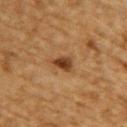Part of a total-body skin-imaging series; this lesion was reviewed on a skin check and was not flagged for biopsy. On the upper back. Approximately 2.5 mm at its widest. The subject is a male approximately 85 years of age or older. Automated tile analysis of the lesion measured a mean CIELAB color near L≈35 a*≈20 b*≈32 and roughly 13 lightness units darker than nearby skin. And it measured a border-irregularity index near 2.5/10, internal color variation of about 3.5 on a 0–10 scale, and peripheral color asymmetry of about 1. Captured under cross-polarized illumination. This image is a 15 mm lesion crop taken from a total-body photograph.